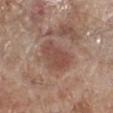| feature | finding |
|---|---|
| biopsy status | imaged on a skin check; not biopsied |
| anatomic site | the left lower leg |
| lesion diameter | about 4 mm |
| acquisition | ~15 mm tile from a whole-body skin photo |
| illumination | white-light |
| patient | male, aged 68 to 72 |
| automated lesion analysis | a footprint of about 10 mm² and an eccentricity of roughly 0.6; a lesion color around L≈48 a*≈21 b*≈25 in CIELAB and about 8 CIELAB-L* units darker than the surrounding skin; an automated nevus-likeness rating near 15 out of 100 and a lesion-detection confidence of about 100/100 |